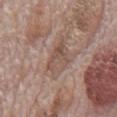Captured during whole-body skin photography for melanoma surveillance; the lesion was not biopsied.
A 15 mm close-up extracted from a 3D total-body photography capture.
On the mid back.
Longest diameter approximately 4.5 mm.
Imaged with white-light lighting.
A male subject aged 68 to 72.
The lesion-visualizer software estimated an area of roughly 9.5 mm² and a shape-asymmetry score of about 0.3 (0 = symmetric). And it measured a mean CIELAB color near L≈51 a*≈17 b*≈25, about 8 CIELAB-L* units darker than the surrounding skin, and a normalized lesion–skin contrast near 5.5. The analysis additionally found border irregularity of about 3.5 on a 0–10 scale and a within-lesion color-variation index near 5/10.